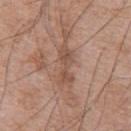biopsy_status: not biopsied; imaged during a skin examination